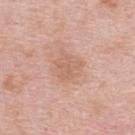Q: Was this lesion biopsied?
A: catalogued during a skin exam; not biopsied
Q: Lesion size?
A: ≈3.5 mm
Q: Where on the body is the lesion?
A: the upper back
Q: How was this image acquired?
A: 15 mm crop, total-body photography
Q: What are the patient's age and sex?
A: female, in their mid- to late 60s
Q: What did automated image analysis measure?
A: an average lesion color of about L≈63 a*≈21 b*≈29 (CIELAB), about 7 CIELAB-L* units darker than the surrounding skin, and a normalized border contrast of about 5; border irregularity of about 2.5 on a 0–10 scale, a color-variation rating of about 2/10, and radial color variation of about 0.5; a classifier nevus-likeness of about 0/100 and a lesion-detection confidence of about 100/100